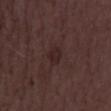This lesion was catalogued during total-body skin photography and was not selected for biopsy. Measured at roughly 2.5 mm in maximum diameter. Imaged with white-light lighting. A female subject, aged around 35. Cropped from a whole-body photographic skin survey; the tile spans about 15 mm. Located on the left thigh.